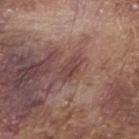Case summary:
* notes · imaged on a skin check; not biopsied
* tile lighting · white-light
* diameter · about 2.5 mm
* automated metrics · a lesion area of about 3 mm², an eccentricity of roughly 0.8, and two-axis asymmetry of about 0.35; a lesion color around L≈42 a*≈21 b*≈22 in CIELAB and a normalized border contrast of about 6
* anatomic site · the right forearm
* subject · male, aged around 75
* image source · ~15 mm tile from a whole-body skin photo